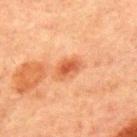site: the chest
automated metrics: an area of roughly 6 mm², an eccentricity of roughly 0.75, and a shape-asymmetry score of about 0.25 (0 = symmetric); a border-irregularity rating of about 2.5/10, a within-lesion color-variation index near 4.5/10, and radial color variation of about 1
subject: male, aged 63 to 67
acquisition: ~15 mm tile from a whole-body skin photo